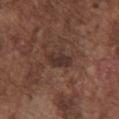Q: Was a biopsy performed?
A: total-body-photography surveillance lesion; no biopsy